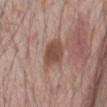illumination — white-light illumination | body site — the abdomen | automated metrics — a footprint of about 8.5 mm², a shape eccentricity near 0.75, and two-axis asymmetry of about 0.25; a classifier nevus-likeness of about 15/100 and lesion-presence confidence of about 100/100 | acquisition — ~15 mm tile from a whole-body skin photo | patient — male, roughly 70 years of age | diameter — about 4 mm.